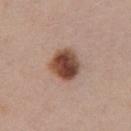Assessment:
Captured during whole-body skin photography for melanoma surveillance; the lesion was not biopsied.
Context:
A female subject aged approximately 45. The tile uses white-light illumination. Located on the chest. A 15 mm close-up tile from a total-body photography series done for melanoma screening.